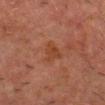Approximately 3 mm at its widest. The lesion is located on the head or neck. Automated tile analysis of the lesion measured a footprint of about 4.5 mm², an eccentricity of roughly 0.65, and two-axis asymmetry of about 0.4. The analysis additionally found a mean CIELAB color near L≈33 a*≈21 b*≈27, a lesion–skin lightness drop of about 5, and a normalized lesion–skin contrast near 6. The software also gave internal color variation of about 2 on a 0–10 scale and peripheral color asymmetry of about 0.5. A 15 mm close-up tile from a total-body photography series done for melanoma screening. A male subject approximately 65 years of age.